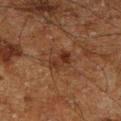  biopsy_status: not biopsied; imaged during a skin examination
  image:
    source: total-body photography crop
    field_of_view_mm: 15
  site: right lower leg
  patient:
    sex: male
    age_approx: 60